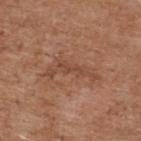Q: What is the anatomic site?
A: the upper back
Q: Automated lesion metrics?
A: a footprint of about 9 mm² and a shape-asymmetry score of about 0.6 (0 = symmetric); an average lesion color of about L≈47 a*≈22 b*≈30 (CIELAB), a lesion–skin lightness drop of about 7, and a lesion-to-skin contrast of about 5.5 (normalized; higher = more distinct)
Q: How was this image acquired?
A: ~15 mm crop, total-body skin-cancer survey
Q: Patient demographics?
A: male, aged approximately 75
Q: What is the lesion's diameter?
A: ~6 mm (longest diameter)
Q: Illumination type?
A: white-light